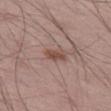This lesion was catalogued during total-body skin photography and was not selected for biopsy. A male subject, in their 70s. The recorded lesion diameter is about 2.5 mm. This is a white-light tile. The lesion-visualizer software estimated two-axis asymmetry of about 0.3. The software also gave a border-irregularity rating of about 3/10 and radial color variation of about 0.5. A roughly 15 mm field-of-view crop from a total-body skin photograph. Located on the leg.